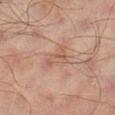Imaged during a routine full-body skin examination; the lesion was not biopsied and no histopathology is available. A 15 mm close-up extracted from a 3D total-body photography capture. The lesion's longest dimension is about 4 mm. A male subject aged 63–67. On the leg. Automated tile analysis of the lesion measured a lesion color around L≈53 a*≈19 b*≈27 in CIELAB, a lesion–skin lightness drop of about 6, and a normalized border contrast of about 5. This is a cross-polarized tile.